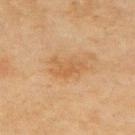This lesion was catalogued during total-body skin photography and was not selected for biopsy. The lesion is on the upper back. Automated tile analysis of the lesion measured an average lesion color of about L≈52 a*≈18 b*≈36 (CIELAB), roughly 6 lightness units darker than nearby skin, and a normalized lesion–skin contrast near 5.5. The analysis additionally found a nevus-likeness score of about 5/100. The tile uses cross-polarized illumination. A female subject, aged approximately 55. Cropped from a total-body skin-imaging series; the visible field is about 15 mm.